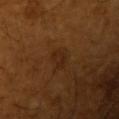Context: A male patient, aged around 65. Imaged with cross-polarized lighting. The lesion-visualizer software estimated a footprint of about 4.5 mm², a shape eccentricity near 0.75, and a symmetry-axis asymmetry near 0.35. The software also gave roughly 5 lightness units darker than nearby skin and a lesion-to-skin contrast of about 5.5 (normalized; higher = more distinct). The analysis additionally found a border-irregularity index near 3.5/10, a within-lesion color-variation index near 3/10, and a peripheral color-asymmetry measure near 1. On the left upper arm. A 15 mm crop from a total-body photograph taken for skin-cancer surveillance.